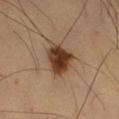Impression:
The lesion was tiled from a total-body skin photograph and was not biopsied.
Context:
Imaged with cross-polarized lighting. Automated image analysis of the tile measured a lesion area of about 11 mm², a shape eccentricity near 0.6, and a shape-asymmetry score of about 0.25 (0 = symmetric). It also reported a mean CIELAB color near L≈38 a*≈19 b*≈30 and roughly 16 lightness units darker than nearby skin. It also reported an automated nevus-likeness rating near 100 out of 100 and lesion-presence confidence of about 100/100. About 4 mm across. A roughly 15 mm field-of-view crop from a total-body skin photograph. A male patient, aged approximately 60. The lesion is on the right thigh.